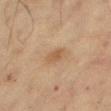Clinical impression: Recorded during total-body skin imaging; not selected for excision or biopsy. Clinical summary: Automated tile analysis of the lesion measured an average lesion color of about L≈48 a*≈17 b*≈31 (CIELAB), roughly 7 lightness units darker than nearby skin, and a lesion-to-skin contrast of about 6 (normalized; higher = more distinct). And it measured a nevus-likeness score of about 45/100. Longest diameter approximately 2.5 mm. A 15 mm close-up extracted from a 3D total-body photography capture. Imaged with cross-polarized lighting. A female subject aged around 55. Located on the right thigh.